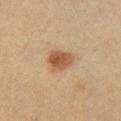Findings:
* lesion diameter: ≈3.5 mm
* acquisition: total-body-photography crop, ~15 mm field of view
* illumination: cross-polarized illumination
* TBP lesion metrics: a lesion area of about 7 mm², a shape eccentricity near 0.55, and a shape-asymmetry score of about 0.25 (0 = symmetric); a within-lesion color-variation index near 4/10 and radial color variation of about 1; an automated nevus-likeness rating near 100 out of 100 and a detector confidence of about 100 out of 100 that the crop contains a lesion
* anatomic site: the chest
* patient: female, roughly 40 years of age